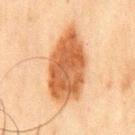This lesion was catalogued during total-body skin photography and was not selected for biopsy. Measured at roughly 9.5 mm in maximum diameter. This image is a 15 mm lesion crop taken from a total-body photograph. A male patient about 45 years old. The lesion is located on the chest.